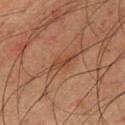workup: imaged on a skin check; not biopsied | patient: male, aged around 35 | site: the chest | image source: total-body-photography crop, ~15 mm field of view.